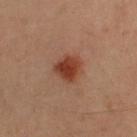Captured during whole-body skin photography for melanoma surveillance; the lesion was not biopsied. This is a cross-polarized tile. A female subject, aged around 30. Located on the back. A 15 mm close-up extracted from a 3D total-body photography capture. Approximately 3.5 mm at its widest.